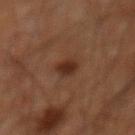• follow-up: catalogued during a skin exam; not biopsied
• location: the mid back
• subject: male, in their 60s
• image source: total-body-photography crop, ~15 mm field of view